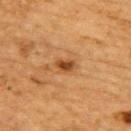Q: Is there a histopathology result?
A: catalogued during a skin exam; not biopsied
Q: What is the imaging modality?
A: ~15 mm crop, total-body skin-cancer survey
Q: Patient demographics?
A: male, about 85 years old or older
Q: Where on the body is the lesion?
A: the upper back
Q: What lighting was used for the tile?
A: cross-polarized illumination
Q: What did automated image analysis measure?
A: a lesion area of about 4 mm² and an outline eccentricity of about 0.8 (0 = round, 1 = elongated); a mean CIELAB color near L≈42 a*≈21 b*≈37 and a normalized border contrast of about 8.5